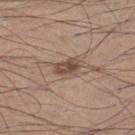Clinical impression:
Recorded during total-body skin imaging; not selected for excision or biopsy.
Clinical summary:
Captured under white-light illumination. A lesion tile, about 15 mm wide, cut from a 3D total-body photograph. The subject is a male aged 33 to 37. On the left lower leg. About 3 mm across.